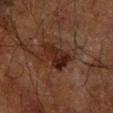Recorded during total-body skin imaging; not selected for excision or biopsy. From the right forearm. A 15 mm close-up extracted from a 3D total-body photography capture. Automated image analysis of the tile measured a lesion color around L≈18 a*≈16 b*≈20 in CIELAB. And it measured a classifier nevus-likeness of about 25/100 and a lesion-detection confidence of about 100/100. The patient is a male about 60 years old. Measured at roughly 5 mm in maximum diameter. This is a cross-polarized tile.